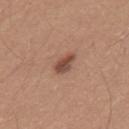biopsy_status: not biopsied; imaged during a skin examination
site: back
patient:
  sex: male
  age_approx: 30
lesion_size:
  long_diameter_mm_approx: 2.5
image:
  source: total-body photography crop
  field_of_view_mm: 15